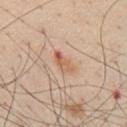{
  "biopsy_status": "not biopsied; imaged during a skin examination",
  "lighting": "white-light",
  "site": "chest",
  "image": {
    "source": "total-body photography crop",
    "field_of_view_mm": 15
  },
  "patient": {
    "sex": "male",
    "age_approx": 60
  },
  "lesion_size": {
    "long_diameter_mm_approx": 3.5
  }
}